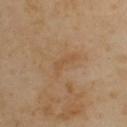The lesion was photographed on a routine skin check and not biopsied; there is no pathology result. Imaged with cross-polarized lighting. A male patient aged approximately 55. The lesion is located on the upper back. The recorded lesion diameter is about 3.5 mm. A region of skin cropped from a whole-body photographic capture, roughly 15 mm wide.